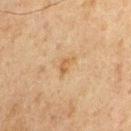The lesion was photographed on a routine skin check and not biopsied; there is no pathology result.
A male patient, in their mid- to late 70s.
Captured under cross-polarized illumination.
Cropped from a whole-body photographic skin survey; the tile spans about 15 mm.
The lesion is located on the chest.
Approximately 2.5 mm at its widest.